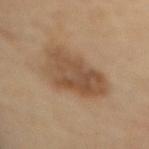| feature | finding |
|---|---|
| follow-up | no biopsy performed (imaged during a skin exam) |
| subject | female, aged around 70 |
| anatomic site | the back |
| imaging modality | total-body-photography crop, ~15 mm field of view |